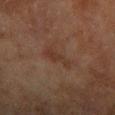Clinical summary: Captured under cross-polarized illumination. A region of skin cropped from a whole-body photographic capture, roughly 15 mm wide. A female patient aged 78–82. From the right forearm.